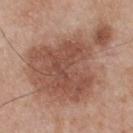{"biopsy_status": "not biopsied; imaged during a skin examination", "site": "upper back", "automated_metrics": {"area_mm2_approx": 48.0, "eccentricity": 0.7, "cielab_L": 50, "cielab_a": 22, "cielab_b": 28, "vs_skin_darker_L": 12.0, "vs_skin_contrast_norm": 8.0, "border_irregularity_0_10": 6.0, "color_variation_0_10": 4.0}, "lesion_size": {"long_diameter_mm_approx": 10.5}, "patient": {"sex": "male", "age_approx": 55}, "image": {"source": "total-body photography crop", "field_of_view_mm": 15}, "lighting": "white-light"}